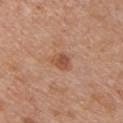follow-up: imaged on a skin check; not biopsied | image: ~15 mm crop, total-body skin-cancer survey | tile lighting: white-light illumination | size: about 2.5 mm | location: the chest | patient: male, in their 60s.